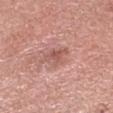biopsy status: total-body-photography surveillance lesion; no biopsy
imaging modality: ~15 mm tile from a whole-body skin photo
body site: the head or neck
illumination: white-light
lesion size: ~3 mm (longest diameter)
TBP lesion metrics: a footprint of about 4.5 mm² and an outline eccentricity of about 0.65 (0 = round, 1 = elongated); roughly 9 lightness units darker than nearby skin and a lesion-to-skin contrast of about 6 (normalized; higher = more distinct); a border-irregularity index near 5/10 and radial color variation of about 0.5; a classifier nevus-likeness of about 0/100
patient: male, aged approximately 60